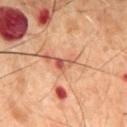No biopsy was performed on this lesion — it was imaged during a full skin examination and was not determined to be concerning.